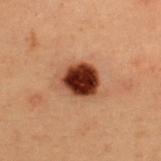biopsy status: imaged on a skin check; not biopsied
imaging modality: ~15 mm tile from a whole-body skin photo
location: the upper back
lighting: cross-polarized illumination
patient: female, aged around 40